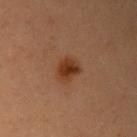| field | value |
|---|---|
| notes | imaged on a skin check; not biopsied |
| subject | female, in their 40s |
| lighting | cross-polarized illumination |
| location | the right upper arm |
| image source | 15 mm crop, total-body photography |
| size | ≈3 mm |